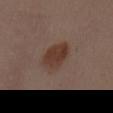workup: imaged on a skin check; not biopsied
illumination: white-light illumination
lesion diameter: about 4.5 mm
body site: the chest
image source: total-body-photography crop, ~15 mm field of view
patient: male, aged 38 to 42